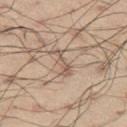<lesion>
  <biopsy_status>not biopsied; imaged during a skin examination</biopsy_status>
</lesion>